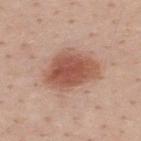This lesion was catalogued during total-body skin photography and was not selected for biopsy.
A roughly 15 mm field-of-view crop from a total-body skin photograph.
This is a white-light tile.
On the upper back.
A male patient, aged around 40.